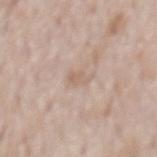| feature | finding |
|---|---|
| notes | total-body-photography surveillance lesion; no biopsy |
| lesion diameter | ~2.5 mm (longest diameter) |
| subject | male, aged 68–72 |
| TBP lesion metrics | a lesion color around L≈62 a*≈15 b*≈26 in CIELAB, a lesion–skin lightness drop of about 7, and a lesion-to-skin contrast of about 4.5 (normalized; higher = more distinct); a border-irregularity index near 3/10, a within-lesion color-variation index near 1.5/10, and a peripheral color-asymmetry measure near 0.5 |
| body site | the lower back |
| lighting | white-light illumination |
| image source | 15 mm crop, total-body photography |